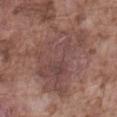Q: Was this lesion biopsied?
A: catalogued during a skin exam; not biopsied
Q: What kind of image is this?
A: 15 mm crop, total-body photography
Q: How was the tile lit?
A: white-light
Q: What are the patient's age and sex?
A: male, aged approximately 75
Q: What did automated image analysis measure?
A: border irregularity of about 6.5 on a 0–10 scale
Q: How large is the lesion?
A: about 9 mm
Q: Lesion location?
A: the abdomen